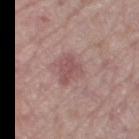Q: Is there a histopathology result?
A: no biopsy performed (imaged during a skin exam)
Q: What is the lesion's diameter?
A: ≈3 mm
Q: Illumination type?
A: white-light illumination
Q: How was this image acquired?
A: ~15 mm crop, total-body skin-cancer survey
Q: What are the patient's age and sex?
A: female, approximately 70 years of age
Q: Where on the body is the lesion?
A: the right thigh